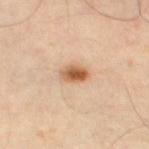Notes:
* imaging modality — ~15 mm crop, total-body skin-cancer survey
* location — the left thigh
* patient — aged around 55
* tile lighting — cross-polarized
* histopathology — an atypical melanocytic neoplasm (borderline)